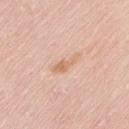- location · the front of the torso
- illumination · white-light illumination
- image source · total-body-photography crop, ~15 mm field of view
- patient · male, about 60 years old